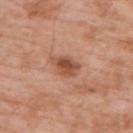Clinical impression:
Part of a total-body skin-imaging series; this lesion was reviewed on a skin check and was not flagged for biopsy.
Background:
The lesion is located on the upper back. A male patient, aged 58 to 62. A roughly 15 mm field-of-view crop from a total-body skin photograph.